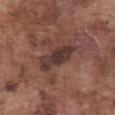{"biopsy_status": "not biopsied; imaged during a skin examination", "image": {"source": "total-body photography crop", "field_of_view_mm": 15}, "patient": {"sex": "male", "age_approx": 75}, "lighting": "white-light", "automated_metrics": {"vs_skin_darker_L": 11.0, "vs_skin_contrast_norm": 10.0, "color_variation_0_10": 3.0, "peripheral_color_asymmetry": 1.0}, "lesion_size": {"long_diameter_mm_approx": 5.5}, "site": "abdomen"}